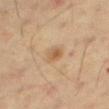Imaged during a routine full-body skin examination; the lesion was not biopsied and no histopathology is available.
A female subject aged around 55.
This is a cross-polarized tile.
The lesion-visualizer software estimated internal color variation of about 4 on a 0–10 scale.
The recorded lesion diameter is about 3 mm.
This image is a 15 mm lesion crop taken from a total-body photograph.
On the right thigh.